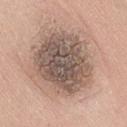image — total-body-photography crop, ~15 mm field of view
subject — male, roughly 75 years of age
lesion diameter — ≈8 mm
site — the lower back